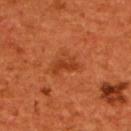Q: Is there a histopathology result?
A: total-body-photography surveillance lesion; no biopsy